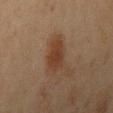No biopsy was performed on this lesion — it was imaged during a full skin examination and was not determined to be concerning.
The lesion is located on the right upper arm.
Cropped from a total-body skin-imaging series; the visible field is about 15 mm.
Longest diameter approximately 4 mm.
The patient is a female in their mid- to late 40s.